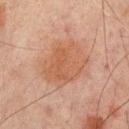No biopsy was performed on this lesion — it was imaged during a full skin examination and was not determined to be concerning.
The total-body-photography lesion software estimated a footprint of about 22 mm², a shape eccentricity near 0.55, and a symmetry-axis asymmetry near 0.2. The analysis additionally found a lesion color around L≈45 a*≈19 b*≈28 in CIELAB and roughly 6 lightness units darker than nearby skin. The software also gave a border-irregularity rating of about 2.5/10, internal color variation of about 3 on a 0–10 scale, and peripheral color asymmetry of about 1. It also reported a nevus-likeness score of about 15/100 and a lesion-detection confidence of about 100/100.
Located on the mid back.
This is a cross-polarized tile.
The subject is a male aged 63 to 67.
A roughly 15 mm field-of-view crop from a total-body skin photograph.